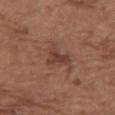{"biopsy_status": "not biopsied; imaged during a skin examination", "site": "chest", "patient": {"sex": "female", "age_approx": 75}, "lighting": "white-light", "lesion_size": {"long_diameter_mm_approx": 3.0}, "image": {"source": "total-body photography crop", "field_of_view_mm": 15}}